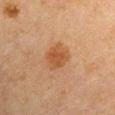The lesion was tiled from a total-body skin photograph and was not biopsied. The lesion is on the left upper arm. A 15 mm crop from a total-body photograph taken for skin-cancer surveillance. The subject is a male about 65 years old.